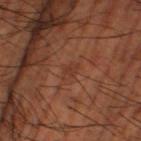<record>
  <biopsy_status>not biopsied; imaged during a skin examination</biopsy_status>
  <lighting>cross-polarized</lighting>
  <image>
    <source>total-body photography crop</source>
    <field_of_view_mm>15</field_of_view_mm>
  </image>
  <lesion_size>
    <long_diameter_mm_approx>2.5</long_diameter_mm_approx>
  </lesion_size>
  <patient>
    <sex>male</sex>
    <age_approx>65</age_approx>
  </patient>
  <site>right thigh</site>
</record>